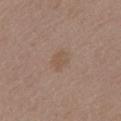No biopsy was performed on this lesion — it was imaged during a full skin examination and was not determined to be concerning. The lesion's longest dimension is about 2.5 mm. A roughly 15 mm field-of-view crop from a total-body skin photograph. This is a white-light tile. On the mid back. The subject is a female roughly 65 years of age.